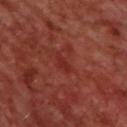On the upper back. The recorded lesion diameter is about 2.5 mm. Captured under cross-polarized illumination. The total-body-photography lesion software estimated a lesion area of about 3 mm², a shape eccentricity near 0.85, and a symmetry-axis asymmetry near 0.35. It also reported a within-lesion color-variation index near 0/10 and peripheral color asymmetry of about 0. And it measured a lesion-detection confidence of about 100/100. A male subject, aged 68–72. A close-up tile cropped from a whole-body skin photograph, about 15 mm across.